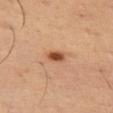<tbp_lesion>
  <biopsy_status>not biopsied; imaged during a skin examination</biopsy_status>
  <automated_metrics>
    <area_mm2_approx>3.5</area_mm2_approx>
    <eccentricity>0.8</eccentricity>
    <shape_asymmetry>0.3</shape_asymmetry>
    <border_irregularity_0_10>2.5</border_irregularity_0_10>
    <color_variation_0_10>3.0</color_variation_0_10>
  </automated_metrics>
  <lesion_size>
    <long_diameter_mm_approx>2.5</long_diameter_mm_approx>
  </lesion_size>
  <patient>
    <sex>male</sex>
    <age_approx>50</age_approx>
  </patient>
  <site>left thigh</site>
  <image>
    <source>total-body photography crop</source>
    <field_of_view_mm>15</field_of_view_mm>
  </image>
</tbp_lesion>